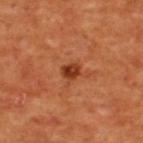Findings:
– notes: imaged on a skin check; not biopsied
– automated lesion analysis: a normalized border contrast of about 9.5; a classifier nevus-likeness of about 85/100
– body site: the upper back
– subject: male, in their mid-50s
– lighting: cross-polarized illumination
– acquisition: 15 mm crop, total-body photography